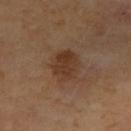<case>
<biopsy_status>not biopsied; imaged during a skin examination</biopsy_status>
<image>
  <source>total-body photography crop</source>
  <field_of_view_mm>15</field_of_view_mm>
</image>
<patient>
  <sex>female</sex>
  <age_approx>40</age_approx>
</patient>
<site>left forearm</site>
<lighting>cross-polarized</lighting>
</case>